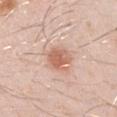biopsy_status: not biopsied; imaged during a skin examination
lighting: white-light
lesion_size:
  long_diameter_mm_approx: 3.5
site: left upper arm
patient:
  sex: male
  age_approx: 30
automated_metrics:
  lesion_detection_confidence_0_100: 100
image:
  source: total-body photography crop
  field_of_view_mm: 15